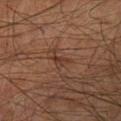biopsy_status: not biopsied; imaged during a skin examination